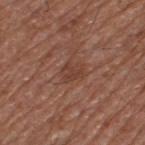Q: Was a biopsy performed?
A: total-body-photography surveillance lesion; no biopsy
Q: How large is the lesion?
A: ~2.5 mm (longest diameter)
Q: Illumination type?
A: white-light illumination
Q: Lesion location?
A: the leg
Q: How was this image acquired?
A: 15 mm crop, total-body photography
Q: Patient demographics?
A: male, approximately 75 years of age
Q: What did automated image analysis measure?
A: roughly 7 lightness units darker than nearby skin and a normalized lesion–skin contrast near 6; a nevus-likeness score of about 0/100 and a detector confidence of about 100 out of 100 that the crop contains a lesion